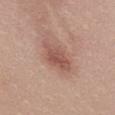{"biopsy_status": "not biopsied; imaged during a skin examination", "image": {"source": "total-body photography crop", "field_of_view_mm": 15}, "lighting": "white-light", "site": "mid back", "patient": {"sex": "female", "age_approx": 20}}